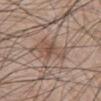Part of a total-body skin-imaging series; this lesion was reviewed on a skin check and was not flagged for biopsy.
A region of skin cropped from a whole-body photographic capture, roughly 15 mm wide.
Longest diameter approximately 4 mm.
A male subject, about 60 years old.
From the front of the torso.
The lesion-visualizer software estimated an automated nevus-likeness rating near 20 out of 100 and lesion-presence confidence of about 100/100.
Captured under white-light illumination.